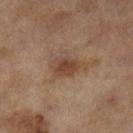Recorded during total-body skin imaging; not selected for excision or biopsy.
The lesion-visualizer software estimated an eccentricity of roughly 0.8. The analysis additionally found internal color variation of about 3.5 on a 0–10 scale and a peripheral color-asymmetry measure near 1. It also reported a nevus-likeness score of about 70/100 and lesion-presence confidence of about 100/100.
The lesion is on the leg.
Imaged with cross-polarized lighting.
A roughly 15 mm field-of-view crop from a total-body skin photograph.
The subject is a female about 60 years old.
Approximately 4 mm at its widest.